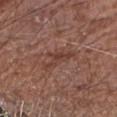Clinical impression: Captured during whole-body skin photography for melanoma surveillance; the lesion was not biopsied. Clinical summary: Captured under white-light illumination. An algorithmic analysis of the crop reported a shape eccentricity near 0.9 and a shape-asymmetry score of about 0.7 (0 = symmetric). It also reported a nevus-likeness score of about 0/100 and a detector confidence of about 85 out of 100 that the crop contains a lesion. A male subject, in their 70s. A 15 mm close-up extracted from a 3D total-body photography capture. The lesion is located on the right forearm. Approximately 4.5 mm at its widest.